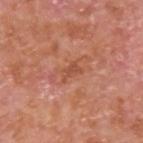A 15 mm close-up tile from a total-body photography series done for melanoma screening. A male subject, aged 63 to 67. Located on the upper back. Imaged with white-light lighting.